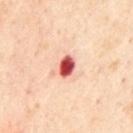Clinical impression: No biopsy was performed on this lesion — it was imaged during a full skin examination and was not determined to be concerning. Acquisition and patient details: Measured at roughly 2.5 mm in maximum diameter. This is a cross-polarized tile. The lesion is located on the chest. Automated tile analysis of the lesion measured a lesion area of about 4.5 mm², an outline eccentricity of about 0.7 (0 = round, 1 = elongated), and two-axis asymmetry of about 0.1. The software also gave a lesion color around L≈58 a*≈38 b*≈31 in CIELAB, about 25 CIELAB-L* units darker than the surrounding skin, and a normalized border contrast of about 14. The software also gave a border-irregularity rating of about 1/10 and internal color variation of about 6 on a 0–10 scale. A 15 mm close-up extracted from a 3D total-body photography capture. A male subject, aged 53–57.